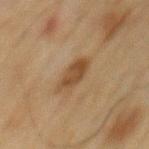Assessment: This lesion was catalogued during total-body skin photography and was not selected for biopsy. Clinical summary: The lesion's longest dimension is about 4.5 mm. The lesion is on the mid back. A close-up tile cropped from a whole-body skin photograph, about 15 mm across. The tile uses cross-polarized illumination. A male patient, aged around 70.